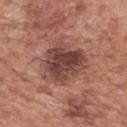patient:
  sex: male
  age_approx: 70
site: mid back
image:
  source: total-body photography crop
  field_of_view_mm: 15